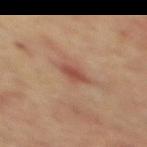Q: Was this lesion biopsied?
A: catalogued during a skin exam; not biopsied
Q: Who is the patient?
A: male, aged 63 to 67
Q: How was the tile lit?
A: cross-polarized illumination
Q: Lesion location?
A: the back
Q: What is the lesion's diameter?
A: about 3 mm
Q: How was this image acquired?
A: ~15 mm tile from a whole-body skin photo